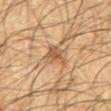Notes:
– workup: no biopsy performed (imaged during a skin exam)
– TBP lesion metrics: a lesion area of about 5 mm²; a lesion color around L≈46 a*≈16 b*≈30 in CIELAB, a lesion–skin lightness drop of about 8, and a normalized border contrast of about 7; a classifier nevus-likeness of about 30/100 and a detector confidence of about 85 out of 100 that the crop contains a lesion
– size: ≈3 mm
– subject: male, roughly 65 years of age
– acquisition: ~15 mm crop, total-body skin-cancer survey
– body site: the abdomen